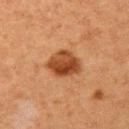Impression:
This lesion was catalogued during total-body skin photography and was not selected for biopsy.
Clinical summary:
This is a cross-polarized tile. On the left upper arm. A 15 mm close-up tile from a total-body photography series done for melanoma screening. A female subject, in their 40s. Longest diameter approximately 4 mm.